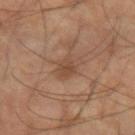Impression:
This lesion was catalogued during total-body skin photography and was not selected for biopsy.
Background:
This is a cross-polarized tile. The subject is a male approximately 60 years of age. Automated tile analysis of the lesion measured a lesion area of about 6 mm², an outline eccentricity of about 0.8 (0 = round, 1 = elongated), and a symmetry-axis asymmetry near 0.25. The analysis additionally found a lesion color around L≈41 a*≈17 b*≈26 in CIELAB, a lesion–skin lightness drop of about 6, and a lesion-to-skin contrast of about 5.5 (normalized; higher = more distinct). The software also gave border irregularity of about 3 on a 0–10 scale and radial color variation of about 0.5. On the arm. This image is a 15 mm lesion crop taken from a total-body photograph.